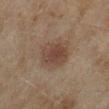follow-up: catalogued during a skin exam; not biopsied
imaging modality: ~15 mm tile from a whole-body skin photo
illumination: cross-polarized illumination
patient: female, in their 60s
location: the left lower leg
image-analysis metrics: a lesion area of about 11 mm², a shape eccentricity near 0.65, and two-axis asymmetry of about 0.15; a border-irregularity index near 1.5/10 and a color-variation rating of about 3.5/10